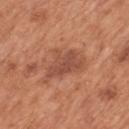<lesion>
  <patient>
    <sex>male</sex>
    <age_approx>65</age_approx>
  </patient>
  <image>
    <source>total-body photography crop</source>
    <field_of_view_mm>15</field_of_view_mm>
  </image>
  <site>mid back</site>
</lesion>